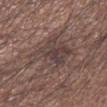No biopsy was performed on this lesion — it was imaged during a full skin examination and was not determined to be concerning.
Longest diameter approximately 5 mm.
The tile uses white-light illumination.
Cropped from a total-body skin-imaging series; the visible field is about 15 mm.
Located on the left forearm.
A male subject aged 63–67.